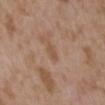The lesion was tiled from a total-body skin photograph and was not biopsied.